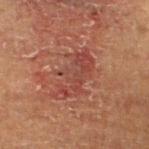Captured during whole-body skin photography for melanoma surveillance; the lesion was not biopsied.